A male subject, aged 68–72 · the lesion is located on the right upper arm · longest diameter approximately 6.5 mm · captured under white-light illumination · this image is a 15 mm lesion crop taken from a total-body photograph: 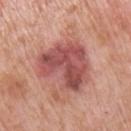– histopathology: an atypical intraepithelial melanocytic proliferation — a borderline lesion of uncertain malignant potential